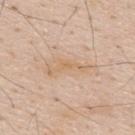notes — no biopsy performed (imaged during a skin exam)
imaging modality — total-body-photography crop, ~15 mm field of view
site — the upper back
tile lighting — white-light illumination
image-analysis metrics — a footprint of about 11 mm² and a shape eccentricity near 0.95; a lesion color around L≈67 a*≈16 b*≈33 in CIELAB, a lesion–skin lightness drop of about 6, and a normalized border contrast of about 5
patient — male, aged around 60